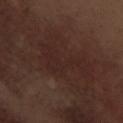Assessment:
This lesion was catalogued during total-body skin photography and was not selected for biopsy.
Background:
The lesion is located on the left forearm. An algorithmic analysis of the crop reported an average lesion color of about L≈24 a*≈17 b*≈19 (CIELAB) and a lesion-to-skin contrast of about 5 (normalized; higher = more distinct). It also reported border irregularity of about 9.5 on a 0–10 scale, internal color variation of about 1 on a 0–10 scale, and a peripheral color-asymmetry measure near 0.5. A region of skin cropped from a whole-body photographic capture, roughly 15 mm wide. A male patient roughly 70 years of age. The tile uses white-light illumination. About 7 mm across.